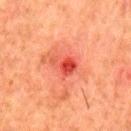Case summary:
– subject · male, about 80 years old
– acquisition · ~15 mm crop, total-body skin-cancer survey
– location · the back